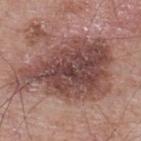{"biopsy_status": "not biopsied; imaged during a skin examination", "lighting": "white-light", "automated_metrics": {"eccentricity": 0.8, "cielab_L": 46, "cielab_a": 21, "cielab_b": 22, "vs_skin_contrast_norm": 10.0, "border_irregularity_0_10": 5.0, "color_variation_0_10": 6.5, "peripheral_color_asymmetry": 2.0}, "patient": {"sex": "male", "age_approx": 65}, "lesion_size": {"long_diameter_mm_approx": 11.0}, "image": {"source": "total-body photography crop", "field_of_view_mm": 15}, "site": "upper back"}